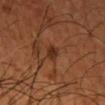Notes:
• notes — total-body-photography surveillance lesion; no biopsy
• acquisition — ~15 mm crop, total-body skin-cancer survey
• subject — male, in their mid- to late 40s
• body site — the head or neck
• illumination — cross-polarized
• lesion diameter — about 2.5 mm